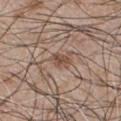The lesion was photographed on a routine skin check and not biopsied; there is no pathology result. A male subject aged approximately 50. A 15 mm crop from a total-body photograph taken for skin-cancer surveillance. The lesion is on the chest.